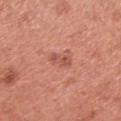Impression:
The lesion was tiled from a total-body skin photograph and was not biopsied.
Context:
A 15 mm close-up tile from a total-body photography series done for melanoma screening. The patient is a female aged approximately 50. Automated image analysis of the tile measured a lesion area of about 3.5 mm², a shape eccentricity near 0.85, and a symmetry-axis asymmetry near 0.3. And it measured a mean CIELAB color near L≈54 a*≈28 b*≈30, a lesion–skin lightness drop of about 9, and a normalized border contrast of about 6. And it measured a lesion-detection confidence of about 100/100. On the arm. Approximately 3 mm at its widest.